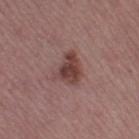{
  "biopsy_status": "not biopsied; imaged during a skin examination",
  "patient": {
    "sex": "female",
    "age_approx": 55
  },
  "image": {
    "source": "total-body photography crop",
    "field_of_view_mm": 15
  },
  "site": "left thigh"
}